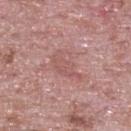Impression:
The lesion was tiled from a total-body skin photograph and was not biopsied.
Background:
The subject is a male aged around 65. This is a white-light tile. The recorded lesion diameter is about 4.5 mm. The lesion is on the back. Automated tile analysis of the lesion measured an eccentricity of roughly 0.8 and a symmetry-axis asymmetry near 0.45. It also reported an average lesion color of about L≈55 a*≈24 b*≈22 (CIELAB), a lesion–skin lightness drop of about 7, and a lesion-to-skin contrast of about 4.5 (normalized; higher = more distinct). A close-up tile cropped from a whole-body skin photograph, about 15 mm across.